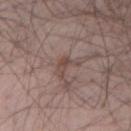biopsy status — total-body-photography surveillance lesion; no biopsy
size — about 3.5 mm
TBP lesion metrics — a lesion area of about 4 mm² and an outline eccentricity of about 0.8 (0 = round, 1 = elongated); a lesion color around L≈46 a*≈16 b*≈21 in CIELAB and a normalized border contrast of about 6; a classifier nevus-likeness of about 0/100 and a lesion-detection confidence of about 95/100
lighting — white-light
imaging modality — ~15 mm tile from a whole-body skin photo
anatomic site — the left forearm
patient — male, in their mid-40s